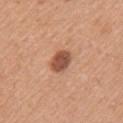Acquisition and patient details:
A 15 mm close-up tile from a total-body photography series done for melanoma screening. The total-body-photography lesion software estimated an average lesion color of about L≈52 a*≈24 b*≈32 (CIELAB), a lesion–skin lightness drop of about 15, and a normalized border contrast of about 10. The recorded lesion diameter is about 3 mm. A female subject aged approximately 35. This is a white-light tile. The lesion is on the arm.